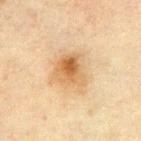biopsy status = total-body-photography surveillance lesion; no biopsy